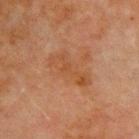patient:
  sex: male
  age_approx: 70
image:
  source: total-body photography crop
  field_of_view_mm: 15
automated_metrics:
  nevus_likeness_0_100: 0
  lesion_detection_confidence_0_100: 100
lesion_size:
  long_diameter_mm_approx: 5.0
site: front of the torso